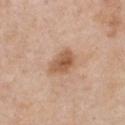Notes:
– follow-up: imaged on a skin check; not biopsied
– body site: the chest
– illumination: white-light
– patient: female, aged 38 to 42
– imaging modality: ~15 mm crop, total-body skin-cancer survey
– lesion diameter: ≈3.5 mm